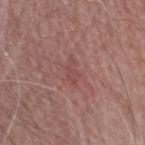Notes:
– notes · total-body-photography surveillance lesion; no biopsy
– patient · male, aged 73 to 77
– imaging modality · total-body-photography crop, ~15 mm field of view
– site · the head or neck
– lesion size · about 3 mm